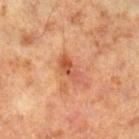Imaged during a routine full-body skin examination; the lesion was not biopsied and no histopathology is available. A 15 mm crop from a total-body photograph taken for skin-cancer surveillance. A male subject roughly 70 years of age. The lesion is located on the right lower leg.